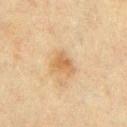notes: no biopsy performed (imaged during a skin exam)
patient: male, aged around 70
image: 15 mm crop, total-body photography
illumination: cross-polarized illumination
site: the chest
TBP lesion metrics: an area of roughly 5 mm² and an outline eccentricity of about 0.5 (0 = round, 1 = elongated); a mean CIELAB color near L≈52 a*≈16 b*≈34, roughly 8 lightness units darker than nearby skin, and a lesion-to-skin contrast of about 6.5 (normalized; higher = more distinct); a border-irregularity rating of about 3.5/10, a color-variation rating of about 2/10, and radial color variation of about 0.5
lesion size: about 2.5 mm